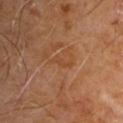  biopsy_status: not biopsied; imaged during a skin examination
  site: upper back
  patient:
    sex: male
    age_approx: 60
  lighting: cross-polarized
  image:
    source: total-body photography crop
    field_of_view_mm: 15